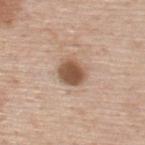The tile uses white-light illumination.
Located on the upper back.
About 3.5 mm across.
A roughly 15 mm field-of-view crop from a total-body skin photograph.
Automated tile analysis of the lesion measured a lesion color around L≈53 a*≈18 b*≈30 in CIELAB, about 15 CIELAB-L* units darker than the surrounding skin, and a normalized border contrast of about 10.
The patient is a male aged 73 to 77.